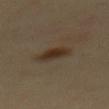Clinical impression: No biopsy was performed on this lesion — it was imaged during a full skin examination and was not determined to be concerning. Context: About 3.5 mm across. A male patient, aged 38–42. Captured under cross-polarized illumination. A roughly 15 mm field-of-view crop from a total-body skin photograph. From the mid back.